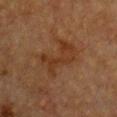Imaged with cross-polarized lighting. Located on the chest. Cropped from a whole-body photographic skin survey; the tile spans about 15 mm. The lesion-visualizer software estimated a footprint of about 12 mm² and a shape eccentricity near 0.85. It also reported a mean CIELAB color near L≈28 a*≈18 b*≈27, a lesion–skin lightness drop of about 6, and a normalized lesion–skin contrast near 6.5. And it measured a nevus-likeness score of about 10/100 and a detector confidence of about 100 out of 100 that the crop contains a lesion. A female patient, aged around 55.